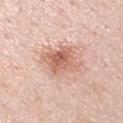The lesion was photographed on a routine skin check and not biopsied; there is no pathology result.
Imaged with white-light lighting.
A close-up tile cropped from a whole-body skin photograph, about 15 mm across.
From the left upper arm.
A male patient, approximately 50 years of age.
Longest diameter approximately 4 mm.
An algorithmic analysis of the crop reported a lesion area of about 12 mm² and an outline eccentricity of about 0.55 (0 = round, 1 = elongated). And it measured border irregularity of about 2.5 on a 0–10 scale, a color-variation rating of about 6/10, and radial color variation of about 2.